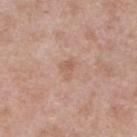Assessment:
Recorded during total-body skin imaging; not selected for excision or biopsy.
Context:
A female patient aged 68 to 72. A 15 mm close-up extracted from a 3D total-body photography capture. The lesion is on the left lower leg.